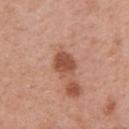| feature | finding |
|---|---|
| notes | total-body-photography surveillance lesion; no biopsy |
| size | ~3.5 mm (longest diameter) |
| tile lighting | white-light illumination |
| patient | female, in their 40s |
| anatomic site | the right upper arm |
| imaging modality | 15 mm crop, total-body photography |
| automated lesion analysis | a border-irregularity index near 2/10 and internal color variation of about 2.5 on a 0–10 scale; an automated nevus-likeness rating near 75 out of 100 and a detector confidence of about 100 out of 100 that the crop contains a lesion |